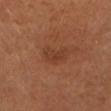Notes:
• biopsy status — no biopsy performed (imaged during a skin exam)
• body site — the head or neck
• automated lesion analysis — a color-variation rating of about 3/10 and radial color variation of about 1
• patient — female, roughly 60 years of age
• illumination — cross-polarized illumination
• lesion size — about 3.5 mm
• acquisition — ~15 mm crop, total-body skin-cancer survey